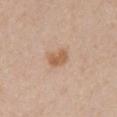Imaged during a routine full-body skin examination; the lesion was not biopsied and no histopathology is available. A female subject, aged approximately 40. Captured under white-light illumination. From the front of the torso. Cropped from a whole-body photographic skin survey; the tile spans about 15 mm. About 2.5 mm across.